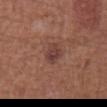| field | value |
|---|---|
| image | 15 mm crop, total-body photography |
| automated lesion analysis | a border-irregularity index near 2.5/10, a color-variation rating of about 3/10, and radial color variation of about 1; an automated nevus-likeness rating near 40 out of 100 and a detector confidence of about 100 out of 100 that the crop contains a lesion |
| size | about 3 mm |
| illumination | white-light |
| subject | male, approximately 65 years of age |
| location | the abdomen |